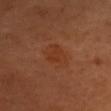| feature | finding |
|---|---|
| workup | catalogued during a skin exam; not biopsied |
| TBP lesion metrics | an area of roughly 7.5 mm² and an eccentricity of roughly 0.8; a mean CIELAB color near L≈28 a*≈20 b*≈27, a lesion–skin lightness drop of about 4, and a lesion-to-skin contrast of about 5 (normalized; higher = more distinct); a nevus-likeness score of about 0/100 and a lesion-detection confidence of about 100/100 |
| anatomic site | the head or neck |
| lighting | cross-polarized illumination |
| lesion size | ~4 mm (longest diameter) |
| image source | ~15 mm tile from a whole-body skin photo |
| patient | male, aged around 55 |